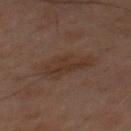Part of a total-body skin-imaging series; this lesion was reviewed on a skin check and was not flagged for biopsy.
A lesion tile, about 15 mm wide, cut from a 3D total-body photograph.
The tile uses cross-polarized illumination.
The lesion is located on the mid back.
About 5 mm across.
The subject is a male aged 58–62.
An algorithmic analysis of the crop reported a lesion area of about 13 mm², an eccentricity of roughly 0.75, and a shape-asymmetry score of about 0.25 (0 = symmetric). And it measured a mean CIELAB color near L≈24 a*≈12 b*≈19, about 4 CIELAB-L* units darker than the surrounding skin, and a normalized border contrast of about 5.5. The software also gave a within-lesion color-variation index near 2/10 and peripheral color asymmetry of about 1.